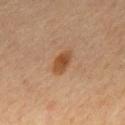follow-up = total-body-photography surveillance lesion; no biopsy
tile lighting = cross-polarized illumination
acquisition = total-body-photography crop, ~15 mm field of view
anatomic site = the mid back
subject = male, in their mid- to late 60s
size = ~3.5 mm (longest diameter)